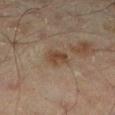No biopsy was performed on this lesion — it was imaged during a full skin examination and was not determined to be concerning. The lesion's longest dimension is about 2.5 mm. Automated image analysis of the tile measured an area of roughly 5 mm² and a shape-asymmetry score of about 0.3 (0 = symmetric). And it measured roughly 8 lightness units darker than nearby skin and a normalized lesion–skin contrast near 7.5. The analysis additionally found a border-irregularity index near 2.5/10, a within-lesion color-variation index near 2.5/10, and a peripheral color-asymmetry measure near 1. The analysis additionally found a classifier nevus-likeness of about 15/100 and a lesion-detection confidence of about 100/100. The lesion is on the right lower leg. The subject is a male aged around 45. Imaged with cross-polarized lighting. A region of skin cropped from a whole-body photographic capture, roughly 15 mm wide.